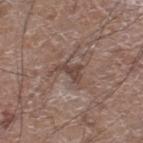Clinical impression: Recorded during total-body skin imaging; not selected for excision or biopsy. Clinical summary: About 3 mm across. A male subject roughly 60 years of age. A 15 mm crop from a total-body photograph taken for skin-cancer surveillance. The lesion is located on the right lower leg. This is a white-light tile.